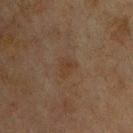workup = no biopsy performed (imaged during a skin exam); subject = male, in their mid- to late 60s; image source = total-body-photography crop, ~15 mm field of view; lesion size = about 2.5 mm; body site = the back; tile lighting = cross-polarized illumination; automated lesion analysis = border irregularity of about 3 on a 0–10 scale and internal color variation of about 2 on a 0–10 scale.